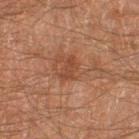Clinical impression: Captured during whole-body skin photography for melanoma surveillance; the lesion was not biopsied. Background: Imaged with cross-polarized lighting. A male subject about 60 years old. A 15 mm close-up tile from a total-body photography series done for melanoma screening. Located on the right thigh.